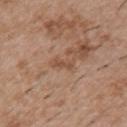Imaged during a routine full-body skin examination; the lesion was not biopsied and no histopathology is available. A male patient, roughly 50 years of age. Captured under white-light illumination. A close-up tile cropped from a whole-body skin photograph, about 15 mm across. About 3 mm across. Automated image analysis of the tile measured a border-irregularity index near 4/10 and internal color variation of about 0 on a 0–10 scale. On the chest.